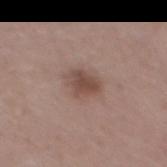Recorded during total-body skin imaging; not selected for excision or biopsy. The lesion's longest dimension is about 3 mm. The patient is a male in their mid- to late 50s. Imaged with white-light lighting. A close-up tile cropped from a whole-body skin photograph, about 15 mm across.